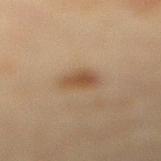Context: Measured at roughly 3 mm in maximum diameter. A close-up tile cropped from a whole-body skin photograph, about 15 mm across. The lesion is on the right lower leg. The tile uses cross-polarized illumination. A female patient in their 40s.